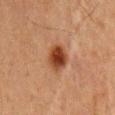Assessment:
This lesion was catalogued during total-body skin photography and was not selected for biopsy.
Context:
The subject is a male in their mid-60s. The tile uses cross-polarized illumination. Cropped from a total-body skin-imaging series; the visible field is about 15 mm. Located on the mid back.